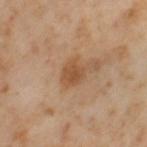Captured during whole-body skin photography for melanoma surveillance; the lesion was not biopsied. Captured under cross-polarized illumination. The lesion is located on the left thigh. An algorithmic analysis of the crop reported a mean CIELAB color near L≈52 a*≈20 b*≈35 and roughly 9 lightness units darker than nearby skin. It also reported a border-irregularity index near 2.5/10 and a peripheral color-asymmetry measure near 1. And it measured a classifier nevus-likeness of about 55/100 and a lesion-detection confidence of about 100/100. The patient is a female in their mid- to late 50s. A 15 mm close-up tile from a total-body photography series done for melanoma screening. The recorded lesion diameter is about 2.5 mm.